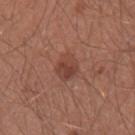Impression:
Recorded during total-body skin imaging; not selected for excision or biopsy.
Image and clinical context:
Automated tile analysis of the lesion measured a footprint of about 6.5 mm², an outline eccentricity of about 0.5 (0 = round, 1 = elongated), and a symmetry-axis asymmetry near 0.2. It also reported about 8 CIELAB-L* units darker than the surrounding skin and a normalized lesion–skin contrast near 6.5. The software also gave an automated nevus-likeness rating near 75 out of 100 and lesion-presence confidence of about 100/100. This image is a 15 mm lesion crop taken from a total-body photograph. A male subject aged 28–32. This is a white-light tile. Measured at roughly 3 mm in maximum diameter. On the right forearm.